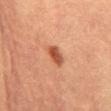Clinical impression: Part of a total-body skin-imaging series; this lesion was reviewed on a skin check and was not flagged for biopsy. Acquisition and patient details: Measured at roughly 3 mm in maximum diameter. Automated image analysis of the tile measured an area of roughly 4 mm², a shape eccentricity near 0.85, and two-axis asymmetry of about 0.25. And it measured a border-irregularity index near 2/10 and radial color variation of about 1. Cropped from a total-body skin-imaging series; the visible field is about 15 mm. This is a cross-polarized tile. Located on the front of the torso. A female patient aged around 70.